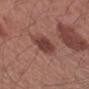Clinical impression:
This lesion was catalogued during total-body skin photography and was not selected for biopsy.
Image and clinical context:
A female subject, aged around 50. The lesion is located on the left forearm. Approximately 3 mm at its widest. A roughly 15 mm field-of-view crop from a total-body skin photograph. Imaged with white-light lighting.